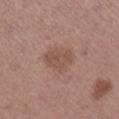{"biopsy_status": "not biopsied; imaged during a skin examination", "site": "leg", "image": {"source": "total-body photography crop", "field_of_view_mm": 15}, "lesion_size": {"long_diameter_mm_approx": 4.0}, "patient": {"sex": "female", "age_approx": 65}}